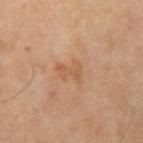<lesion>
  <biopsy_status>not biopsied; imaged during a skin examination</biopsy_status>
  <lighting>cross-polarized</lighting>
  <lesion_size>
    <long_diameter_mm_approx>3.0</long_diameter_mm_approx>
  </lesion_size>
  <site>arm</site>
  <patient>
    <sex>male</sex>
    <age_approx>65</age_approx>
  </patient>
  <image>
    <source>total-body photography crop</source>
    <field_of_view_mm>15</field_of_view_mm>
  </image>
</lesion>